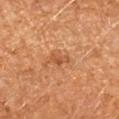{"biopsy_status": "not biopsied; imaged during a skin examination", "lighting": "cross-polarized", "patient": {"sex": "female", "age_approx": 65}, "lesion_size": {"long_diameter_mm_approx": 2.5}, "image": {"source": "total-body photography crop", "field_of_view_mm": 15}, "automated_metrics": {"area_mm2_approx": 3.0, "nevus_likeness_0_100": 5, "lesion_detection_confidence_0_100": 100}, "site": "arm"}